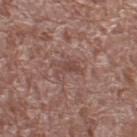Assessment:
Recorded during total-body skin imaging; not selected for excision or biopsy.
Acquisition and patient details:
On the left thigh. A roughly 15 mm field-of-view crop from a total-body skin photograph. A female patient, in their mid-70s. Captured under white-light illumination. About 3 mm across.